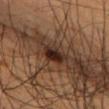biopsy_status: not biopsied; imaged during a skin examination
site: back
lighting: cross-polarized
patient:
  sex: male
  age_approx: 50
lesion_size:
  long_diameter_mm_approx: 3.5
image:
  source: total-body photography crop
  field_of_view_mm: 15
automated_metrics:
  vs_skin_darker_L: 12.0
  vs_skin_contrast_norm: 12.5
  nevus_likeness_0_100: 90
  lesion_detection_confidence_0_100: 100